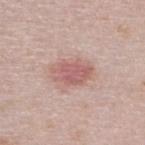Assessment:
The lesion was tiled from a total-body skin photograph and was not biopsied.
Context:
A male subject, in their 40s. This image is a 15 mm lesion crop taken from a total-body photograph. From the upper back.